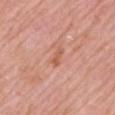This lesion was catalogued during total-body skin photography and was not selected for biopsy. A male subject, roughly 60 years of age. About 2.5 mm across. From the left upper arm. Captured under white-light illumination. A roughly 15 mm field-of-view crop from a total-body skin photograph.